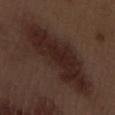The lesion was photographed on a routine skin check and not biopsied; there is no pathology result. A male subject about 70 years old. A 15 mm crop from a total-body photograph taken for skin-cancer surveillance. The lesion is on the left forearm.